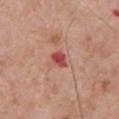follow-up = catalogued during a skin exam; not biopsied | anatomic site = the chest | lesion diameter = ≈2.5 mm | subject = male, roughly 65 years of age | imaging modality = ~15 mm crop, total-body skin-cancer survey | lighting = white-light | image-analysis metrics = about 13 CIELAB-L* units darker than the surrounding skin and a normalized lesion–skin contrast near 9; a border-irregularity rating of about 2/10, a within-lesion color-variation index near 4/10, and peripheral color asymmetry of about 1.5; an automated nevus-likeness rating near 0 out of 100 and a lesion-detection confidence of about 100/100.